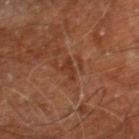Automated image analysis of the tile measured a footprint of about 3 mm², a shape eccentricity near 0.9, and a shape-asymmetry score of about 0.45 (0 = symmetric). The software also gave a lesion color around L≈34 a*≈23 b*≈30 in CIELAB, roughly 7 lightness units darker than nearby skin, and a normalized lesion–skin contrast near 6.5. The analysis additionally found border irregularity of about 5 on a 0–10 scale, a color-variation rating of about 0.5/10, and radial color variation of about 0. The software also gave a nevus-likeness score of about 0/100 and lesion-presence confidence of about 100/100.
The lesion is located on the right leg.
The subject is a male aged 58–62.
Imaged with cross-polarized lighting.
A close-up tile cropped from a whole-body skin photograph, about 15 mm across.